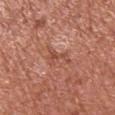No biopsy was performed on this lesion — it was imaged during a full skin examination and was not determined to be concerning. Automated image analysis of the tile measured a lesion area of about 4 mm², an eccentricity of roughly 0.85, and a symmetry-axis asymmetry near 0.65. It also reported a lesion–skin lightness drop of about 8 and a normalized lesion–skin contrast near 6. Cropped from a total-body skin-imaging series; the visible field is about 15 mm. The tile uses white-light illumination. From the right upper arm. A male subject aged 63–67.